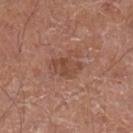Impression: Captured during whole-body skin photography for melanoma surveillance; the lesion was not biopsied.